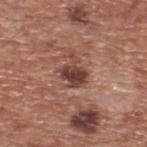Q: Was a biopsy performed?
A: total-body-photography surveillance lesion; no biopsy
Q: What lighting was used for the tile?
A: white-light illumination
Q: Where on the body is the lesion?
A: the upper back
Q: Automated lesion metrics?
A: a lesion color around L≈42 a*≈23 b*≈24 in CIELAB and a normalized lesion–skin contrast near 10; a border-irregularity index near 4/10, a color-variation rating of about 4.5/10, and a peripheral color-asymmetry measure near 1.5; a classifier nevus-likeness of about 40/100 and lesion-presence confidence of about 100/100
Q: How was this image acquired?
A: ~15 mm crop, total-body skin-cancer survey
Q: Patient demographics?
A: male, aged 73–77
Q: Lesion size?
A: about 3.5 mm